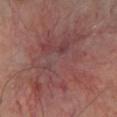A male subject, in their mid- to late 60s.
A 15 mm crop from a total-body photograph taken for skin-cancer surveillance.
From the left lower leg.